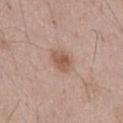notes: catalogued during a skin exam; not biopsied | subject: male, aged 53–57 | site: the abdomen | tile lighting: white-light | size: about 3 mm | automated metrics: a footprint of about 5 mm², an outline eccentricity of about 0.75 (0 = round, 1 = elongated), and two-axis asymmetry of about 0.2; a nevus-likeness score of about 85/100 and a lesion-detection confidence of about 100/100 | acquisition: ~15 mm crop, total-body skin-cancer survey.